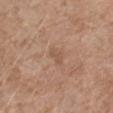{
  "biopsy_status": "not biopsied; imaged during a skin examination"
}